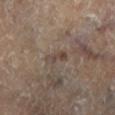Clinical impression:
Imaged during a routine full-body skin examination; the lesion was not biopsied and no histopathology is available.